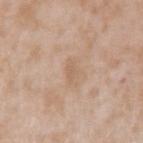Findings:
- notes · imaged on a skin check; not biopsied
- automated lesion analysis · border irregularity of about 4.5 on a 0–10 scale, internal color variation of about 0 on a 0–10 scale, and radial color variation of about 0; a nevus-likeness score of about 0/100
- site · the left upper arm
- image · ~15 mm crop, total-body skin-cancer survey
- subject · male, aged around 45
- tile lighting · white-light illumination